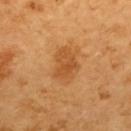Assessment: No biopsy was performed on this lesion — it was imaged during a full skin examination and was not determined to be concerning. Image and clinical context: Automated image analysis of the tile measured a shape-asymmetry score of about 0.25 (0 = symmetric). It also reported about 9 CIELAB-L* units darker than the surrounding skin and a lesion-to-skin contrast of about 6.5 (normalized; higher = more distinct). It also reported border irregularity of about 2.5 on a 0–10 scale and a peripheral color-asymmetry measure near 1. The patient is a female aged around 55. Cropped from a total-body skin-imaging series; the visible field is about 15 mm. Located on the back.